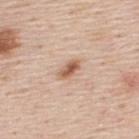<tbp_lesion>
<biopsy_status>not biopsied; imaged during a skin examination</biopsy_status>
<image>
  <source>total-body photography crop</source>
  <field_of_view_mm>15</field_of_view_mm>
</image>
<lighting>white-light</lighting>
<patient>
  <sex>male</sex>
  <age_approx>45</age_approx>
</patient>
<site>upper back</site>
<lesion_size>
  <long_diameter_mm_approx>2.5</long_diameter_mm_approx>
</lesion_size>
</tbp_lesion>